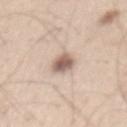size: ≈3.5 mm
subject: male, aged around 60
image: ~15 mm crop, total-body skin-cancer survey
tile lighting: white-light
body site: the back
automated lesion analysis: a shape eccentricity near 0.75; an average lesion color of about L≈61 a*≈16 b*≈25 (CIELAB), about 15 CIELAB-L* units darker than the surrounding skin, and a normalized lesion–skin contrast near 9.5; radial color variation of about 2